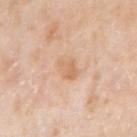A 15 mm close-up tile from a total-body photography series done for melanoma screening.
A male subject, aged approximately 75.
On the right upper arm.